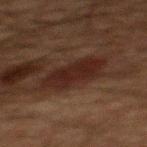Q: Was a biopsy performed?
A: no biopsy performed (imaged during a skin exam)
Q: What are the patient's age and sex?
A: male, aged around 60
Q: What is the imaging modality?
A: total-body-photography crop, ~15 mm field of view
Q: What is the anatomic site?
A: the mid back
Q: Automated lesion metrics?
A: a peripheral color-asymmetry measure near 1
Q: How was the tile lit?
A: cross-polarized illumination